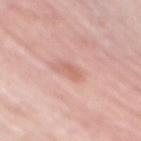The lesion's longest dimension is about 3 mm. A female subject, approximately 60 years of age. This image is a 15 mm lesion crop taken from a total-body photograph. An algorithmic analysis of the crop reported an average lesion color of about L≈63 a*≈24 b*≈27 (CIELAB), about 8 CIELAB-L* units darker than the surrounding skin, and a normalized border contrast of about 5.5. The analysis additionally found peripheral color asymmetry of about 0. The lesion is located on the mid back. This is a white-light tile.